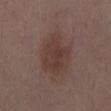The tile uses white-light illumination. The lesion is on the chest. Cropped from a whole-body photographic skin survey; the tile spans about 15 mm. The total-body-photography lesion software estimated an average lesion color of about L≈37 a*≈17 b*≈22 (CIELAB), about 7 CIELAB-L* units darker than the surrounding skin, and a normalized lesion–skin contrast near 7. The analysis additionally found a color-variation rating of about 3/10 and a peripheral color-asymmetry measure near 1. It also reported a nevus-likeness score of about 20/100 and lesion-presence confidence of about 100/100. A female subject, aged 28–32. The lesion's longest dimension is about 6 mm.